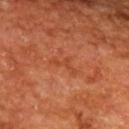Recorded during total-body skin imaging; not selected for excision or biopsy.
A lesion tile, about 15 mm wide, cut from a 3D total-body photograph.
Imaged with cross-polarized lighting.
On the upper back.
A male patient, in their mid- to late 60s.